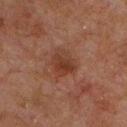– biopsy status — total-body-photography surveillance lesion; no biopsy
– site — the chest
– lesion size — ≈3.5 mm
– image — total-body-photography crop, ~15 mm field of view
– illumination — cross-polarized
– subject — male, aged approximately 60